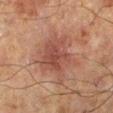Assessment: Recorded during total-body skin imaging; not selected for excision or biopsy. Image and clinical context: The patient is a male aged 63–67. This is a cross-polarized tile. Approximately 6.5 mm at its widest. The lesion is on the right lower leg. This image is a 15 mm lesion crop taken from a total-body photograph.